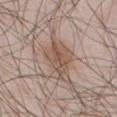<tbp_lesion>
  <biopsy_status>not biopsied; imaged during a skin examination</biopsy_status>
  <image>
    <source>total-body photography crop</source>
    <field_of_view_mm>15</field_of_view_mm>
  </image>
  <patient>
    <sex>male</sex>
    <age_approx>55</age_approx>
  </patient>
  <lighting>white-light</lighting>
  <lesion_size>
    <long_diameter_mm_approx>4.0</long_diameter_mm_approx>
  </lesion_size>
  <site>abdomen</site>
</tbp_lesion>